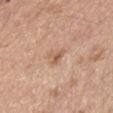This lesion was catalogued during total-body skin photography and was not selected for biopsy. Cropped from a total-body skin-imaging series; the visible field is about 15 mm. A male subject aged around 70. The lesion is on the abdomen.